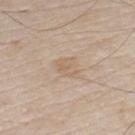Findings:
– follow-up — catalogued during a skin exam; not biopsied
– subject — male, approximately 80 years of age
– body site — the chest
– imaging modality — ~15 mm crop, total-body skin-cancer survey